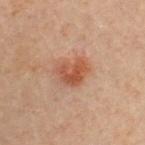Recorded during total-body skin imaging; not selected for excision or biopsy. Approximately 4 mm at its widest. Located on the chest. The tile uses cross-polarized illumination. A lesion tile, about 15 mm wide, cut from a 3D total-body photograph. A female patient in their 30s.